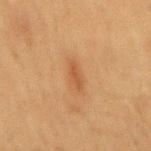Acquisition and patient details:
On the mid back. This is a cross-polarized tile. The lesion's longest dimension is about 3 mm. The subject is a male aged 58 to 62. Cropped from a whole-body photographic skin survey; the tile spans about 15 mm. The lesion-visualizer software estimated a lesion area of about 3.5 mm², an eccentricity of roughly 0.95, and a shape-asymmetry score of about 0.25 (0 = symmetric). And it measured a lesion color around L≈45 a*≈21 b*≈34 in CIELAB and a normalized lesion–skin contrast near 6. And it measured a classifier nevus-likeness of about 90/100 and a detector confidence of about 100 out of 100 that the crop contains a lesion.